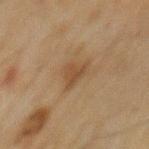biopsy status: no biopsy performed (imaged during a skin exam) | image-analysis metrics: a lesion color around L≈38 a*≈15 b*≈29 in CIELAB, roughly 7 lightness units darker than nearby skin, and a lesion-to-skin contrast of about 6.5 (normalized; higher = more distinct); a border-irregularity rating of about 5.5/10, internal color variation of about 1.5 on a 0–10 scale, and a peripheral color-asymmetry measure near 0.5; lesion-presence confidence of about 100/100 | anatomic site: the mid back | imaging modality: ~15 mm crop, total-body skin-cancer survey | subject: male, roughly 70 years of age.